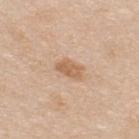This lesion was catalogued during total-body skin photography and was not selected for biopsy.
On the upper back.
Imaged with white-light lighting.
A roughly 15 mm field-of-view crop from a total-body skin photograph.
The lesion's longest dimension is about 3 mm.
The subject is a male in their mid-30s.